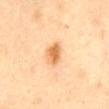biopsy status: catalogued during a skin exam; not biopsied.